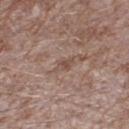No biopsy was performed on this lesion — it was imaged during a full skin examination and was not determined to be concerning. A 15 mm close-up tile from a total-body photography series done for melanoma screening. About 2.5 mm across. The tile uses white-light illumination. On the right thigh. The subject is a male about 45 years old.